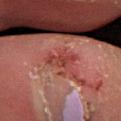workup = total-body-photography surveillance lesion; no biopsy | tile lighting = cross-polarized | patient = female, in their 50s | body site = the right lower leg | image-analysis metrics = an area of roughly 4 mm² and two-axis asymmetry of about 0.4; a lesion color around L≈33 a*≈26 b*≈24 in CIELAB, roughly 6 lightness units darker than nearby skin, and a normalized lesion–skin contrast near 6.5; border irregularity of about 5 on a 0–10 scale, a color-variation rating of about 2/10, and radial color variation of about 0.5 | imaging modality = total-body-photography crop, ~15 mm field of view | lesion size = ~3.5 mm (longest diameter).